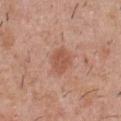Notes:
- biopsy status — no biopsy performed (imaged during a skin exam)
- subject — male, about 30 years old
- body site — the front of the torso
- image — 15 mm crop, total-body photography
- automated lesion analysis — an outline eccentricity of about 0.7 (0 = round, 1 = elongated) and two-axis asymmetry of about 0.2; an average lesion color of about L≈54 a*≈24 b*≈31 (CIELAB) and a lesion–skin lightness drop of about 9; a border-irregularity rating of about 2/10, a within-lesion color-variation index near 2/10, and a peripheral color-asymmetry measure near 1; a classifier nevus-likeness of about 45/100